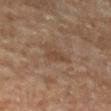Clinical impression:
The lesion was photographed on a routine skin check and not biopsied; there is no pathology result.
Context:
A female subject aged around 70. The lesion is on the left lower leg. A 15 mm close-up extracted from a 3D total-body photography capture.